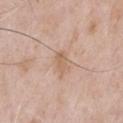{"site": "chest", "lesion_size": {"long_diameter_mm_approx": 2.5}, "patient": {"sex": "male", "age_approx": 50}, "lighting": "white-light", "image": {"source": "total-body photography crop", "field_of_view_mm": 15}}